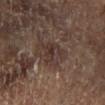Part of a total-body skin-imaging series; this lesion was reviewed on a skin check and was not flagged for biopsy.
The patient is a male aged around 65.
The lesion-visualizer software estimated a lesion area of about 8 mm² and a symmetry-axis asymmetry near 0.35. It also reported an average lesion color of about L≈31 a*≈13 b*≈19 (CIELAB) and a normalized lesion–skin contrast near 6.5. The software also gave a nevus-likeness score of about 5/100 and a lesion-detection confidence of about 90/100.
On the left lower leg.
Imaged with cross-polarized lighting.
A close-up tile cropped from a whole-body skin photograph, about 15 mm across.
The lesion's longest dimension is about 3.5 mm.